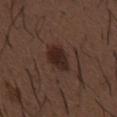Impression:
Recorded during total-body skin imaging; not selected for excision or biopsy.
Image and clinical context:
A male patient, aged approximately 50. A 15 mm close-up extracted from a 3D total-body photography capture. Automated tile analysis of the lesion measured an outline eccentricity of about 0.8 (0 = round, 1 = elongated) and two-axis asymmetry of about 0.15. The analysis additionally found internal color variation of about 3.5 on a 0–10 scale and peripheral color asymmetry of about 1. It also reported a nevus-likeness score of about 90/100 and a lesion-detection confidence of about 100/100. Located on the abdomen. Imaged with white-light lighting.